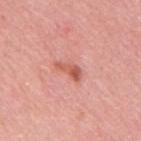The lesion was photographed on a routine skin check and not biopsied; there is no pathology result. A male patient roughly 50 years of age. This is a white-light tile. The lesion is located on the mid back. Automated image analysis of the tile measured a footprint of about 4.5 mm², a shape eccentricity near 0.9, and two-axis asymmetry of about 0.35. And it measured an automated nevus-likeness rating near 20 out of 100 and a detector confidence of about 100 out of 100 that the crop contains a lesion. The recorded lesion diameter is about 3.5 mm. A lesion tile, about 15 mm wide, cut from a 3D total-body photograph.